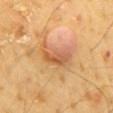Recorded during total-body skin imaging; not selected for excision or biopsy.
Captured under cross-polarized illumination.
The patient is a male approximately 65 years of age.
A 15 mm close-up extracted from a 3D total-body photography capture.
Longest diameter approximately 4.5 mm.
From the back.